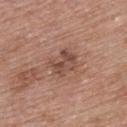Impression: Part of a total-body skin-imaging series; this lesion was reviewed on a skin check and was not flagged for biopsy. Background: Approximately 3.5 mm at its widest. Located on the upper back. Cropped from a whole-body photographic skin survey; the tile spans about 15 mm. Captured under white-light illumination. The patient is a female about 50 years old.